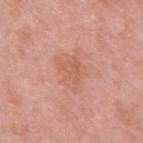This lesion was catalogued during total-body skin photography and was not selected for biopsy. From the upper back. Longest diameter approximately 4.5 mm. Cropped from a whole-body photographic skin survey; the tile spans about 15 mm. Imaged with white-light lighting. The patient is a male roughly 40 years of age.